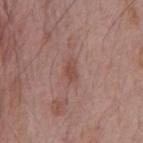The lesion was tiled from a total-body skin photograph and was not biopsied. The recorded lesion diameter is about 2.5 mm. A male subject, approximately 70 years of age. Captured under white-light illumination. On the chest. A lesion tile, about 15 mm wide, cut from a 3D total-body photograph.